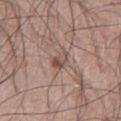Q: What is the lesion's diameter?
A: ≈2.5 mm
Q: What is the anatomic site?
A: the left thigh
Q: What is the imaging modality?
A: ~15 mm tile from a whole-body skin photo
Q: Illumination type?
A: white-light illumination
Q: Patient demographics?
A: male, aged approximately 55